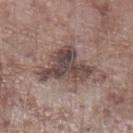follow-up: no biopsy performed (imaged during a skin exam) | subject: male, aged approximately 75 | automated metrics: an area of roughly 17 mm², an outline eccentricity of about 0.7 (0 = round, 1 = elongated), and two-axis asymmetry of about 0.55; a mean CIELAB color near L≈45 a*≈15 b*≈18, roughly 13 lightness units darker than nearby skin, and a lesion-to-skin contrast of about 10 (normalized; higher = more distinct); a within-lesion color-variation index near 5.5/10 and radial color variation of about 2; a classifier nevus-likeness of about 0/100 and a detector confidence of about 95 out of 100 that the crop contains a lesion | anatomic site: the left lower leg | lighting: white-light | size: ≈6.5 mm | image source: ~15 mm crop, total-body skin-cancer survey.